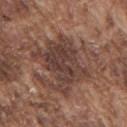Findings:
• image · ~15 mm crop, total-body skin-cancer survey
• patient · male, aged 73 to 77
• lesion diameter · ≈9.5 mm
• tile lighting · white-light illumination
• site · the mid back